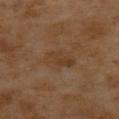Q: Is there a histopathology result?
A: total-body-photography surveillance lesion; no biopsy
Q: What is the imaging modality?
A: 15 mm crop, total-body photography
Q: What is the anatomic site?
A: the upper back
Q: Who is the patient?
A: female, in their mid- to late 50s
Q: Illumination type?
A: cross-polarized illumination